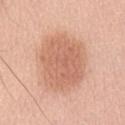Case summary:
- workup — catalogued during a skin exam; not biopsied
- image — ~15 mm crop, total-body skin-cancer survey
- patient — female, aged approximately 65
- body site — the lower back